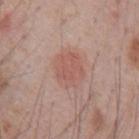biopsy status — no biopsy performed (imaged during a skin exam); subject — male, aged 68–72; site — the chest; image — total-body-photography crop, ~15 mm field of view.